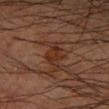workup: catalogued during a skin exam; not biopsied
TBP lesion metrics: a shape-asymmetry score of about 0.55 (0 = symmetric); a lesion color around L≈22 a*≈18 b*≈23 in CIELAB and a lesion–skin lightness drop of about 6; internal color variation of about 0 on a 0–10 scale and a peripheral color-asymmetry measure near 0
site: the right forearm
imaging modality: 15 mm crop, total-body photography
subject: male, in their mid- to late 60s
lesion diameter: ≈2.5 mm
illumination: cross-polarized